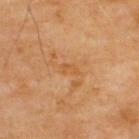Q: Was this lesion biopsied?
A: catalogued during a skin exam; not biopsied
Q: Automated lesion metrics?
A: a lesion–skin lightness drop of about 6 and a normalized lesion–skin contrast near 5; a border-irregularity index near 4/10 and radial color variation of about 0
Q: What is the anatomic site?
A: the upper back
Q: What kind of image is this?
A: total-body-photography crop, ~15 mm field of view
Q: Who is the patient?
A: male, approximately 70 years of age
Q: Lesion size?
A: ~2.5 mm (longest diameter)
Q: How was the tile lit?
A: cross-polarized